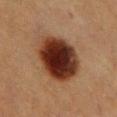Recorded during total-body skin imaging; not selected for excision or biopsy.
From the chest.
A 15 mm close-up tile from a total-body photography series done for melanoma screening.
This is a cross-polarized tile.
The subject is a female aged 38 to 42.